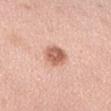Q: How was the tile lit?
A: white-light illumination
Q: What is the anatomic site?
A: the right forearm
Q: What is the lesion's diameter?
A: ≈3 mm
Q: What kind of image is this?
A: total-body-photography crop, ~15 mm field of view
Q: Patient demographics?
A: female, about 50 years old